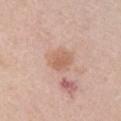<lesion>
  <biopsy_status>not biopsied; imaged during a skin examination</biopsy_status>
  <lesion_size>
    <long_diameter_mm_approx>3.0</long_diameter_mm_approx>
  </lesion_size>
  <site>front of the torso</site>
  <image>
    <source>total-body photography crop</source>
    <field_of_view_mm>15</field_of_view_mm>
  </image>
  <lighting>white-light</lighting>
  <patient>
    <sex>female</sex>
    <age_approx>60</age_approx>
  </patient>
</lesion>